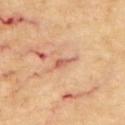Q: Was a biopsy performed?
A: total-body-photography surveillance lesion; no biopsy
Q: Where on the body is the lesion?
A: the upper back
Q: Patient demographics?
A: male, about 70 years old
Q: What is the imaging modality?
A: 15 mm crop, total-body photography